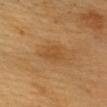| feature | finding |
|---|---|
| patient | male, about 60 years old |
| anatomic site | the head or neck |
| size | ≈3 mm |
| lighting | cross-polarized illumination |
| image source | 15 mm crop, total-body photography |